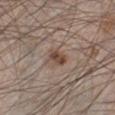No biopsy was performed on this lesion — it was imaged during a full skin examination and was not determined to be concerning.
The patient is a male roughly 60 years of age.
A lesion tile, about 15 mm wide, cut from a 3D total-body photograph.
Automated tile analysis of the lesion measured a footprint of about 4.5 mm². The software also gave a mean CIELAB color near L≈46 a*≈16 b*≈25 and a lesion-to-skin contrast of about 8.5 (normalized; higher = more distinct). And it measured a classifier nevus-likeness of about 80/100 and a lesion-detection confidence of about 100/100.
The lesion is on the left lower leg.
The tile uses white-light illumination.